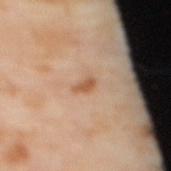Clinical impression: Imaged during a routine full-body skin examination; the lesion was not biopsied and no histopathology is available. Context: This image is a 15 mm lesion crop taken from a total-body photograph. The subject is a female in their 50s. On the mid back.